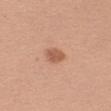Clinical impression: The lesion was photographed on a routine skin check and not biopsied; there is no pathology result. Acquisition and patient details: The tile uses white-light illumination. Located on the left upper arm. Automated tile analysis of the lesion measured a border-irregularity rating of about 2/10, a within-lesion color-variation index near 1.5/10, and radial color variation of about 0.5. A close-up tile cropped from a whole-body skin photograph, about 15 mm across. The patient is a female aged 43–47.